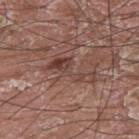| field | value |
|---|---|
| notes | total-body-photography surveillance lesion; no biopsy |
| lighting | white-light |
| location | the upper back |
| subject | male, roughly 40 years of age |
| image source | ~15 mm tile from a whole-body skin photo |
| TBP lesion metrics | an automated nevus-likeness rating near 0 out of 100 and lesion-presence confidence of about 85/100 |
| diameter | about 6 mm |